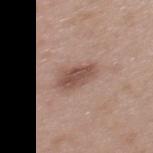Findings:
• workup · catalogued during a skin exam; not biopsied
• site · the upper back
• lesion diameter · about 4 mm
• image source · 15 mm crop, total-body photography
• subject · female, roughly 40 years of age
• image-analysis metrics · a lesion area of about 7 mm²; a border-irregularity index near 2/10 and a within-lesion color-variation index near 2.5/10
• illumination · white-light illumination